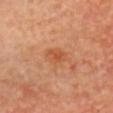Impression: Captured during whole-body skin photography for melanoma surveillance; the lesion was not biopsied.